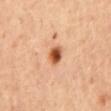body site=the mid back; image source=15 mm crop, total-body photography; subject=female, in their mid- to late 40s; diameter=~2.5 mm (longest diameter); tile lighting=cross-polarized.